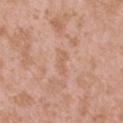| key | value |
|---|---|
| follow-up | catalogued during a skin exam; not biopsied |
| location | the upper back |
| lighting | white-light |
| image | ~15 mm tile from a whole-body skin photo |
| subject | female, roughly 40 years of age |
| automated metrics | a color-variation rating of about 0.5/10 and radial color variation of about 0; a nevus-likeness score of about 0/100 and a lesion-detection confidence of about 95/100 |